The subject is a female approximately 50 years of age · a roughly 15 mm field-of-view crop from a total-body skin photograph: 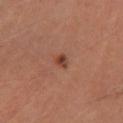{
  "lesion_size": {
    "long_diameter_mm_approx": 1.5
  },
  "lighting": "cross-polarized",
  "diagnosis": {
    "histopathology": "junctional melanocytic nevus",
    "malignancy": "benign",
    "taxonomic_path": [
      "Benign",
      "Benign melanocytic proliferations",
      "Nevus",
      "Nevus, NOS, Junctional"
    ]
  }
}